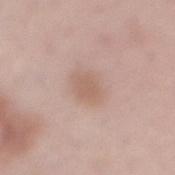Impression: No biopsy was performed on this lesion — it was imaged during a full skin examination and was not determined to be concerning. Clinical summary: A male patient, aged 53–57. Automated image analysis of the tile measured a mean CIELAB color near L≈61 a*≈18 b*≈27, about 7 CIELAB-L* units darker than the surrounding skin, and a normalized lesion–skin contrast near 5.5. The recorded lesion diameter is about 3 mm. The tile uses white-light illumination. Cropped from a total-body skin-imaging series; the visible field is about 15 mm. The lesion is located on the mid back.